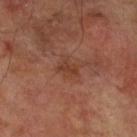<tbp_lesion>
  <biopsy_status>not biopsied; imaged during a skin examination</biopsy_status>
  <lighting>cross-polarized</lighting>
  <lesion_size>
    <long_diameter_mm_approx>2.5</long_diameter_mm_approx>
  </lesion_size>
  <automated_metrics>
    <area_mm2_approx>3.0</area_mm2_approx>
    <eccentricity>0.8</eccentricity>
    <shape_asymmetry>0.4</shape_asymmetry>
    <cielab_L>38</cielab_L>
    <cielab_a>23</cielab_a>
    <cielab_b>30</cielab_b>
    <vs_skin_darker_L>7.0</vs_skin_darker_L>
    <vs_skin_contrast_norm>6.5</vs_skin_contrast_norm>
    <nevus_likeness_0_100>0</nevus_likeness_0_100>
    <lesion_detection_confidence_0_100>100</lesion_detection_confidence_0_100>
  </automated_metrics>
  <site>right lower leg</site>
  <patient>
    <sex>male</sex>
    <age_approx>70</age_approx>
  </patient>
  <image>
    <source>total-body photography crop</source>
    <field_of_view_mm>15</field_of_view_mm>
  </image>
</tbp_lesion>